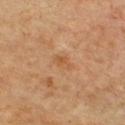{
  "biopsy_status": "not biopsied; imaged during a skin examination",
  "image": {
    "source": "total-body photography crop",
    "field_of_view_mm": 15
  },
  "lesion_size": {
    "long_diameter_mm_approx": 2.5
  },
  "automated_metrics": {
    "area_mm2_approx": 3.5,
    "border_irregularity_0_10": 2.5,
    "color_variation_0_10": 2.0,
    "peripheral_color_asymmetry": 0.5,
    "nevus_likeness_0_100": 0,
    "lesion_detection_confidence_0_100": 100
  },
  "site": "chest",
  "patient": {
    "sex": "male",
    "age_approx": 60
  }
}